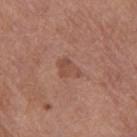No biopsy was performed on this lesion — it was imaged during a full skin examination and was not determined to be concerning.
Longest diameter approximately 3 mm.
The lesion is on the leg.
A female subject, aged 63–67.
Imaged with white-light lighting.
A region of skin cropped from a whole-body photographic capture, roughly 15 mm wide.